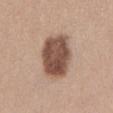This lesion was catalogued during total-body skin photography and was not selected for biopsy.
The tile uses white-light illumination.
Cropped from a total-body skin-imaging series; the visible field is about 15 mm.
The lesion's longest dimension is about 5.5 mm.
A female patient, aged 38 to 42.
The lesion is located on the back.